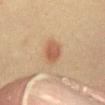biopsy_status: not biopsied; imaged during a skin examination
patient:
  sex: male
  age_approx: 40
image:
  source: total-body photography crop
  field_of_view_mm: 15
lighting: cross-polarized
site: abdomen
lesion_size:
  long_diameter_mm_approx: 3.5